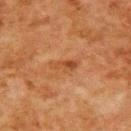Q: Is there a histopathology result?
A: total-body-photography surveillance lesion; no biopsy
Q: What kind of image is this?
A: ~15 mm tile from a whole-body skin photo
Q: Lesion location?
A: the upper back
Q: Who is the patient?
A: male, roughly 80 years of age